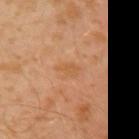This lesion was catalogued during total-body skin photography and was not selected for biopsy. A male patient roughly 30 years of age. Cropped from a whole-body photographic skin survey; the tile spans about 15 mm. From the left upper arm.